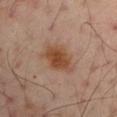Impression:
Recorded during total-body skin imaging; not selected for excision or biopsy.
Background:
Longest diameter approximately 4.5 mm. A 15 mm close-up tile from a total-body photography series done for melanoma screening. A male subject, aged 48 to 52. On the left arm. Automated tile analysis of the lesion measured an average lesion color of about L≈46 a*≈20 b*≈31 (CIELAB), about 10 CIELAB-L* units darker than the surrounding skin, and a normalized lesion–skin contrast near 9. The analysis additionally found border irregularity of about 3 on a 0–10 scale, a within-lesion color-variation index near 4/10, and peripheral color asymmetry of about 1. The tile uses cross-polarized illumination.